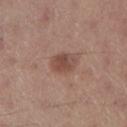follow-up — imaged on a skin check; not biopsied
tile lighting — white-light illumination
automated metrics — a footprint of about 6 mm², a shape eccentricity near 0.6, and a symmetry-axis asymmetry near 0.15; roughly 10 lightness units darker than nearby skin and a normalized lesion–skin contrast near 7.5; lesion-presence confidence of about 100/100
patient — male, roughly 55 years of age
size — about 3 mm
acquisition — ~15 mm crop, total-body skin-cancer survey
anatomic site — the right lower leg